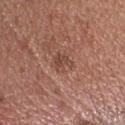Captured during whole-body skin photography for melanoma surveillance; the lesion was not biopsied. A region of skin cropped from a whole-body photographic capture, roughly 15 mm wide. The lesion is on the head or neck. The patient is a male aged 53 to 57. The tile uses white-light illumination. The lesion's longest dimension is about 2.5 mm. Automated tile analysis of the lesion measured a lesion-to-skin contrast of about 6 (normalized; higher = more distinct). The analysis additionally found a border-irregularity index near 4.5/10.